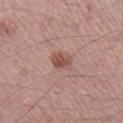A 15 mm crop from a total-body photograph taken for skin-cancer surveillance. Imaged with white-light lighting. On the right lower leg. A male patient, aged approximately 55. An algorithmic analysis of the crop reported a detector confidence of about 100 out of 100 that the crop contains a lesion. Measured at roughly 3 mm in maximum diameter.